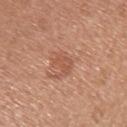Clinical summary:
A female subject, aged approximately 50. The tile uses white-light illumination. The lesion is on the left upper arm. About 2.5 mm across. A 15 mm close-up tile from a total-body photography series done for melanoma screening.